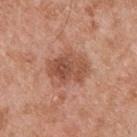patient:
  sex: male
  age_approx: 55
image:
  source: total-body photography crop
  field_of_view_mm: 15
site: upper back